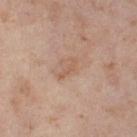workup = total-body-photography surveillance lesion; no biopsy | patient = female, in their mid-50s | lesion diameter = ≈3 mm | illumination = cross-polarized | imaging modality = ~15 mm tile from a whole-body skin photo | location = the left thigh | automated lesion analysis = an area of roughly 2.5 mm², an eccentricity of roughly 0.95, and two-axis asymmetry of about 0.45; a border-irregularity rating of about 5.5/10, internal color variation of about 0 on a 0–10 scale, and radial color variation of about 0; a classifier nevus-likeness of about 0/100.